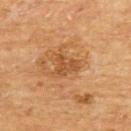The total-body-photography lesion software estimated border irregularity of about 6.5 on a 0–10 scale and a peripheral color-asymmetry measure near 0.5. The software also gave a classifier nevus-likeness of about 20/100 and a lesion-detection confidence of about 100/100.
The tile uses cross-polarized illumination.
A 15 mm close-up extracted from a 3D total-body photography capture.
A male subject, aged 63 to 67.
The lesion is on the upper back.
Approximately 3 mm at its widest.